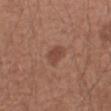biopsy_status: not biopsied; imaged during a skin examination
lesion_size:
  long_diameter_mm_approx: 3.0
patient:
  sex: male
  age_approx: 50
lighting: white-light
site: arm
image:
  source: total-body photography crop
  field_of_view_mm: 15